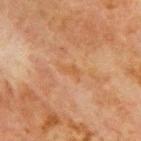Impression:
Captured during whole-body skin photography for melanoma surveillance; the lesion was not biopsied.
Acquisition and patient details:
A close-up tile cropped from a whole-body skin photograph, about 15 mm across. Imaged with cross-polarized lighting. Automated image analysis of the tile measured an eccentricity of roughly 0.9. The software also gave a lesion color around L≈46 a*≈19 b*≈33 in CIELAB and a lesion-to-skin contrast of about 5 (normalized; higher = more distinct). The analysis additionally found a border-irregularity index near 5.5/10, a within-lesion color-variation index near 0.5/10, and radial color variation of about 0. And it measured an automated nevus-likeness rating near 0 out of 100 and a detector confidence of about 100 out of 100 that the crop contains a lesion. The lesion is on the front of the torso. A male subject, aged 63–67.